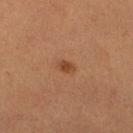{
  "patient": {
    "sex": "female",
    "age_approx": 40
  },
  "lesion_size": {
    "long_diameter_mm_approx": 2.5
  },
  "site": "right lower leg",
  "image": {
    "source": "total-body photography crop",
    "field_of_view_mm": 15
  }
}